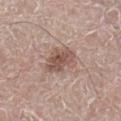{"biopsy_status": "not biopsied; imaged during a skin examination", "patient": {"sex": "male", "age_approx": 65}, "lesion_size": {"long_diameter_mm_approx": 4.5}, "lighting": "white-light", "image": {"source": "total-body photography crop", "field_of_view_mm": 15}, "site": "right lower leg", "automated_metrics": {"vs_skin_darker_L": 11.0, "vs_skin_contrast_norm": 8.0, "border_irregularity_0_10": 3.0, "color_variation_0_10": 4.0, "peripheral_color_asymmetry": 1.0, "nevus_likeness_0_100": 85, "lesion_detection_confidence_0_100": 100}}